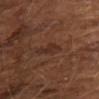notes: no biopsy performed (imaged during a skin exam)
size: about 2.5 mm
subject: male, about 65 years old
image source: 15 mm crop, total-body photography
illumination: cross-polarized
image-analysis metrics: a lesion color around L≈28 a*≈18 b*≈24 in CIELAB, about 5 CIELAB-L* units darker than the surrounding skin, and a normalized lesion–skin contrast near 6; a within-lesion color-variation index near 1.5/10; an automated nevus-likeness rating near 0 out of 100